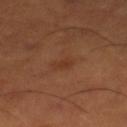Case summary:
- workup: imaged on a skin check; not biopsied
- body site: the right lower leg
- subject: male, aged approximately 50
- imaging modality: total-body-photography crop, ~15 mm field of view
- lesion size: ≈3 mm